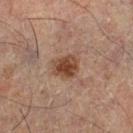Imaged during a routine full-body skin examination; the lesion was not biopsied and no histopathology is available. The tile uses cross-polarized illumination. Approximately 3.5 mm at its widest. Automated tile analysis of the lesion measured an average lesion color of about L≈39 a*≈19 b*≈27 (CIELAB), roughly 11 lightness units darker than nearby skin, and a lesion-to-skin contrast of about 10 (normalized; higher = more distinct). It also reported border irregularity of about 2.5 on a 0–10 scale and radial color variation of about 1.5. And it measured an automated nevus-likeness rating near 95 out of 100 and lesion-presence confidence of about 100/100. Cropped from a whole-body photographic skin survey; the tile spans about 15 mm. A male patient, roughly 50 years of age. The lesion is on the leg.